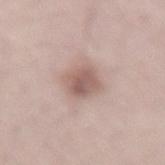Assessment: Part of a total-body skin-imaging series; this lesion was reviewed on a skin check and was not flagged for biopsy. Clinical summary: The lesion is located on the back. The recorded lesion diameter is about 3.5 mm. A 15 mm crop from a total-body photograph taken for skin-cancer surveillance. A male subject, about 50 years old.